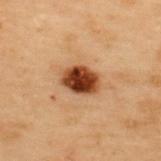Impression:
Part of a total-body skin-imaging series; this lesion was reviewed on a skin check and was not flagged for biopsy.
Context:
The lesion's longest dimension is about 4 mm. The tile uses cross-polarized illumination. A male patient aged approximately 55. The lesion is located on the upper back. A 15 mm crop from a total-body photograph taken for skin-cancer surveillance.